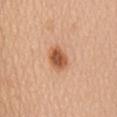The lesion was tiled from a total-body skin photograph and was not biopsied.
Automated image analysis of the tile measured an area of roughly 7 mm², a shape eccentricity near 0.7, and a symmetry-axis asymmetry near 0.15. The analysis additionally found a lesion color around L≈57 a*≈25 b*≈37 in CIELAB.
The tile uses white-light illumination.
Located on the mid back.
A 15 mm close-up tile from a total-body photography series done for melanoma screening.
Measured at roughly 3.5 mm in maximum diameter.
A female subject, about 70 years old.